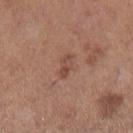{
  "lesion_size": {
    "long_diameter_mm_approx": 3.0
  },
  "automated_metrics": {
    "area_mm2_approx": 3.5,
    "eccentricity": 0.9,
    "shape_asymmetry": 0.25,
    "border_irregularity_0_10": 2.5,
    "color_variation_0_10": 4.0,
    "peripheral_color_asymmetry": 1.5,
    "nevus_likeness_0_100": 10,
    "lesion_detection_confidence_0_100": 100
  },
  "image": {
    "source": "total-body photography crop",
    "field_of_view_mm": 15
  },
  "lighting": "white-light",
  "patient": {
    "sex": "female",
    "age_approx": 55
  },
  "site": "left lower leg"
}